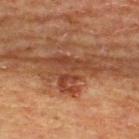biopsy status — catalogued during a skin exam; not biopsied